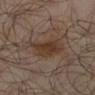• follow-up — total-body-photography surveillance lesion; no biopsy
• patient — male, aged 63 to 67
• location — the leg
• TBP lesion metrics — an area of roughly 12 mm², an eccentricity of roughly 0.75, and two-axis asymmetry of about 0.2
• size — ≈4.5 mm
• imaging modality — total-body-photography crop, ~15 mm field of view
• lighting — cross-polarized